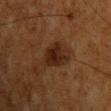Context: A male patient, roughly 60 years of age. The lesion is located on the front of the torso. The lesion's longest dimension is about 4 mm. Cropped from a whole-body photographic skin survey; the tile spans about 15 mm.